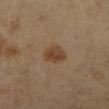Assessment:
Imaged during a routine full-body skin examination; the lesion was not biopsied and no histopathology is available.
Image and clinical context:
Located on the leg. Longest diameter approximately 3 mm. Captured under cross-polarized illumination. The patient is a female aged 58–62. A region of skin cropped from a whole-body photographic capture, roughly 15 mm wide.